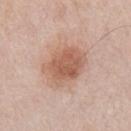Captured during whole-body skin photography for melanoma surveillance; the lesion was not biopsied.
A male subject roughly 75 years of age.
The lesion is on the chest.
The tile uses white-light illumination.
A close-up tile cropped from a whole-body skin photograph, about 15 mm across.
Longest diameter approximately 5.5 mm.